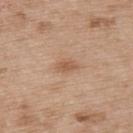The lesion was photographed on a routine skin check and not biopsied; there is no pathology result.
A male subject in their 50s.
A region of skin cropped from a whole-body photographic capture, roughly 15 mm wide.
On the upper back.